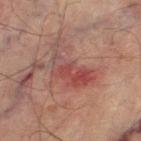Clinical summary: A male patient, in their mid-70s. Cropped from a total-body skin-imaging series; the visible field is about 15 mm. The lesion is on the leg. Automated image analysis of the tile measured a lesion area of about 14 mm², an eccentricity of roughly 0.85, and two-axis asymmetry of about 0.5. It also reported a mean CIELAB color near L≈40 a*≈23 b*≈21, about 7 CIELAB-L* units darker than the surrounding skin, and a normalized lesion–skin contrast near 6.5. It also reported border irregularity of about 7 on a 0–10 scale, internal color variation of about 6.5 on a 0–10 scale, and radial color variation of about 2.5. About 6 mm across. Imaged with cross-polarized lighting.